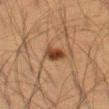| field | value |
|---|---|
| workup | catalogued during a skin exam; not biopsied |
| tile lighting | cross-polarized illumination |
| location | the leg |
| image | ~15 mm crop, total-body skin-cancer survey |
| patient | male, roughly 65 years of age |
| lesion size | about 3 mm |